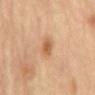{"biopsy_status": "not biopsied; imaged during a skin examination", "site": "mid back", "lighting": "cross-polarized", "automated_metrics": {"shape_asymmetry": 0.3, "border_irregularity_0_10": 3.0, "color_variation_0_10": 3.5, "peripheral_color_asymmetry": 1.0}, "lesion_size": {"long_diameter_mm_approx": 2.5}, "image": {"source": "total-body photography crop", "field_of_view_mm": 15}, "patient": {"sex": "male", "age_approx": "85+"}}